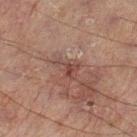Q: Was this lesion biopsied?
A: catalogued during a skin exam; not biopsied
Q: How was this image acquired?
A: 15 mm crop, total-body photography
Q: Lesion location?
A: the left lower leg
Q: What is the lesion's diameter?
A: ≈3 mm
Q: What are the patient's age and sex?
A: male, aged around 60
Q: Automated lesion metrics?
A: an area of roughly 5 mm², a shape eccentricity near 0.7, and two-axis asymmetry of about 0.55; a lesion color around L≈33 a*≈16 b*≈18 in CIELAB, roughly 6 lightness units darker than nearby skin, and a normalized lesion–skin contrast near 6; a border-irregularity index near 5/10 and radial color variation of about 1; an automated nevus-likeness rating near 0 out of 100 and lesion-presence confidence of about 95/100